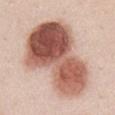The lesion was tiled from a total-body skin photograph and was not biopsied.
A roughly 15 mm field-of-view crop from a total-body skin photograph.
Located on the chest.
Longest diameter approximately 11.5 mm.
The total-body-photography lesion software estimated a footprint of about 55 mm², a shape eccentricity near 0.85, and two-axis asymmetry of about 0.4. It also reported about 20 CIELAB-L* units darker than the surrounding skin and a normalized lesion–skin contrast near 12.5.
A male subject, aged around 25.